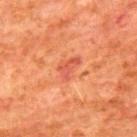notes: no biopsy performed (imaged during a skin exam)
location: the upper back
imaging modality: total-body-photography crop, ~15 mm field of view
subject: male, aged 78–82